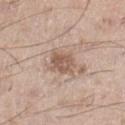Case summary:
* biopsy status — catalogued during a skin exam; not biopsied
* diameter — ≈3 mm
* image-analysis metrics — an area of roughly 6.5 mm², an outline eccentricity of about 0.3 (0 = round, 1 = elongated), and a symmetry-axis asymmetry near 0.15; border irregularity of about 2 on a 0–10 scale, internal color variation of about 3.5 on a 0–10 scale, and a peripheral color-asymmetry measure near 1.5
* image source — ~15 mm tile from a whole-body skin photo
* subject — male, aged around 35
* body site — the left lower leg
* tile lighting — white-light illumination